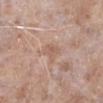Notes:
- biopsy status · catalogued during a skin exam; not biopsied
- lesion diameter · about 2.5 mm
- imaging modality · 15 mm crop, total-body photography
- lighting · white-light
- subject · male, about 75 years old
- location · the right lower leg